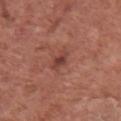biopsy status = imaged on a skin check; not biopsied | tile lighting = white-light | imaging modality = total-body-photography crop, ~15 mm field of view | anatomic site = the chest | patient = male, aged 73–77.